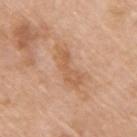This lesion was catalogued during total-body skin photography and was not selected for biopsy. The subject is a female in their mid- to late 70s. The lesion's longest dimension is about 5 mm. On the right upper arm. The total-body-photography lesion software estimated an area of roughly 8.5 mm², a shape eccentricity near 0.9, and two-axis asymmetry of about 0.35. The software also gave border irregularity of about 4.5 on a 0–10 scale and radial color variation of about 1. The analysis additionally found a lesion-detection confidence of about 100/100. A close-up tile cropped from a whole-body skin photograph, about 15 mm across.